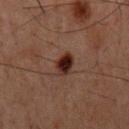Imaged during a routine full-body skin examination; the lesion was not biopsied and no histopathology is available. From the mid back. This image is a 15 mm lesion crop taken from a total-body photograph. A male subject about 60 years old.